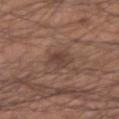This lesion was catalogued during total-body skin photography and was not selected for biopsy. The subject is a male aged around 55. Captured under white-light illumination. A lesion tile, about 15 mm wide, cut from a 3D total-body photograph. From the right forearm. Longest diameter approximately 3 mm. The total-body-photography lesion software estimated lesion-presence confidence of about 100/100.